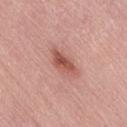Q: Was a biopsy performed?
A: catalogued during a skin exam; not biopsied
Q: What is the imaging modality?
A: 15 mm crop, total-body photography
Q: What is the anatomic site?
A: the lower back
Q: What are the patient's age and sex?
A: female, about 65 years old
Q: Illumination type?
A: white-light illumination